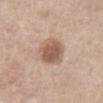{
  "automated_metrics": {
    "area_mm2_approx": 12.0,
    "eccentricity": 0.55,
    "shape_asymmetry": 0.15,
    "vs_skin_contrast_norm": 8.5,
    "lesion_detection_confidence_0_100": 100
  },
  "patient": {
    "sex": "female",
    "age_approx": 65
  },
  "image": {
    "source": "total-body photography crop",
    "field_of_view_mm": 15
  },
  "lesion_size": {
    "long_diameter_mm_approx": 4.0
  },
  "site": "abdomen",
  "lighting": "white-light"
}